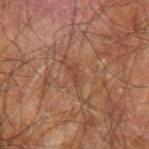No biopsy was performed on this lesion — it was imaged during a full skin examination and was not determined to be concerning. Imaged with cross-polarized lighting. From the right forearm. A 15 mm close-up tile from a total-body photography series done for melanoma screening. A male patient aged 68 to 72. Automated tile analysis of the lesion measured a mean CIELAB color near L≈35 a*≈19 b*≈26, a lesion–skin lightness drop of about 5, and a normalized lesion–skin contrast near 5. The lesion's longest dimension is about 2.5 mm.